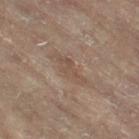From the left leg.
An algorithmic analysis of the crop reported a lesion color around L≈44 a*≈14 b*≈23 in CIELAB, about 6 CIELAB-L* units darker than the surrounding skin, and a lesion-to-skin contrast of about 5 (normalized; higher = more distinct). The software also gave a classifier nevus-likeness of about 0/100 and lesion-presence confidence of about 70/100.
A female patient, aged around 80.
Cropped from a total-body skin-imaging series; the visible field is about 15 mm.
About 4.5 mm across.